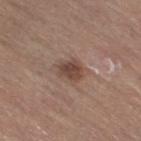Context:
A roughly 15 mm field-of-view crop from a total-body skin photograph. Captured under white-light illumination. The lesion is located on the right thigh. A female subject, aged 63 to 67. About 3 mm across.